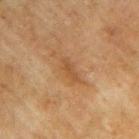| field | value |
|---|---|
| biopsy status | imaged on a skin check; not biopsied |
| TBP lesion metrics | an area of roughly 3.5 mm² and an eccentricity of roughly 0.9; an automated nevus-likeness rating near 0 out of 100 |
| subject | male, approximately 75 years of age |
| location | the right forearm |
| lesion diameter | ≈3.5 mm |
| tile lighting | cross-polarized illumination |
| image source | ~15 mm crop, total-body skin-cancer survey |